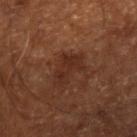Recorded during total-body skin imaging; not selected for excision or biopsy.